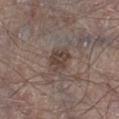Clinical summary: Longest diameter approximately 3 mm. A male subject, in their mid-60s. Imaged with white-light lighting. A region of skin cropped from a whole-body photographic capture, roughly 15 mm wide. The lesion is on the left lower leg.